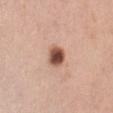Image and clinical context: A region of skin cropped from a whole-body photographic capture, roughly 15 mm wide. A female patient in their 50s. On the abdomen.